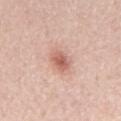No biopsy was performed on this lesion — it was imaged during a full skin examination and was not determined to be concerning.